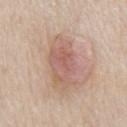follow-up = imaged on a skin check; not biopsied
image source = 15 mm crop, total-body photography
patient = male, approximately 65 years of age
lighting = white-light illumination
lesion diameter = ≈7 mm
body site = the chest
image-analysis metrics = an area of roughly 18 mm² and two-axis asymmetry of about 0.2; a mean CIELAB color near L≈60 a*≈20 b*≈26, roughly 10 lightness units darker than nearby skin, and a normalized border contrast of about 6.5